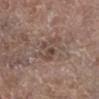notes=catalogued during a skin exam; not biopsied | tile lighting=white-light illumination | image-analysis metrics=roughly 7 lightness units darker than nearby skin; a nevus-likeness score of about 0/100 and a detector confidence of about 100 out of 100 that the crop contains a lesion | site=the left lower leg | size=about 4 mm | patient=male, aged approximately 65 | imaging modality=~15 mm crop, total-body skin-cancer survey.